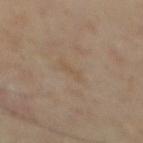The lesion was photographed on a routine skin check and not biopsied; there is no pathology result. A close-up tile cropped from a whole-body skin photograph, about 15 mm across. The lesion is located on the mid back. The lesion-visualizer software estimated an area of roughly 2.5 mm² and a shape-asymmetry score of about 0.4 (0 = symmetric). The analysis additionally found a classifier nevus-likeness of about 0/100 and a lesion-detection confidence of about 100/100. Longest diameter approximately 3 mm. A male patient, aged around 65.